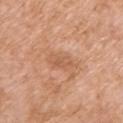Located on the arm. Measured at roughly 3.5 mm in maximum diameter. A 15 mm close-up tile from a total-body photography series done for melanoma screening. The tile uses white-light illumination. A female subject, about 75 years old.